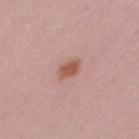automated lesion analysis: a lesion area of about 4 mm² and an outline eccentricity of about 0.75 (0 = round, 1 = elongated); a lesion color around L≈55 a*≈24 b*≈27 in CIELAB, roughly 11 lightness units darker than nearby skin, and a lesion-to-skin contrast of about 8.5 (normalized; higher = more distinct); a nevus-likeness score of about 95/100
patient: female, aged approximately 45
site: the right upper arm
illumination: white-light illumination
image source: ~15 mm crop, total-body skin-cancer survey